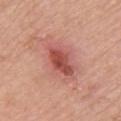Case summary:
- image — total-body-photography crop, ~15 mm field of view
- tile lighting — white-light
- subject — female, roughly 65 years of age
- site — the upper back